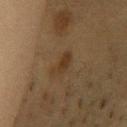No biopsy was performed on this lesion — it was imaged during a full skin examination and was not determined to be concerning. Longest diameter approximately 3.5 mm. The patient is a female aged approximately 40. The lesion-visualizer software estimated two-axis asymmetry of about 0.3. It also reported border irregularity of about 3.5 on a 0–10 scale and internal color variation of about 2 on a 0–10 scale. It also reported a classifier nevus-likeness of about 15/100 and a detector confidence of about 100 out of 100 that the crop contains a lesion. The lesion is on the chest. A 15 mm close-up extracted from a 3D total-body photography capture.